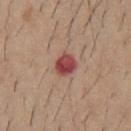Automated tile analysis of the lesion measured an area of roughly 6 mm² and an eccentricity of roughly 0.45.
The lesion's longest dimension is about 3 mm.
A roughly 15 mm field-of-view crop from a total-body skin photograph.
On the chest.
Captured under white-light illumination.
The subject is a male roughly 60 years of age.